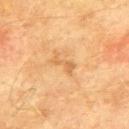<lesion>
  <biopsy_status>not biopsied; imaged during a skin examination</biopsy_status>
  <patient>
    <sex>male</sex>
    <age_approx>75</age_approx>
  </patient>
  <lighting>cross-polarized</lighting>
  <automated_metrics>
    <eccentricity>0.9</eccentricity>
    <shape_asymmetry>0.45</shape_asymmetry>
    <border_irregularity_0_10>5.5</border_irregularity_0_10>
    <color_variation_0_10>0.5</color_variation_0_10>
    <peripheral_color_asymmetry>0.0</peripheral_color_asymmetry>
  </automated_metrics>
  <lesion_size>
    <long_diameter_mm_approx>3.0</long_diameter_mm_approx>
  </lesion_size>
  <site>back</site>
  <image>
    <source>total-body photography crop</source>
    <field_of_view_mm>15</field_of_view_mm>
  </image>
</lesion>